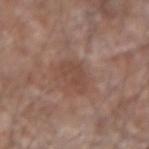Notes:
* notes: catalogued during a skin exam; not biopsied
* lighting: white-light illumination
* patient: male, about 60 years old
* anatomic site: the arm
* automated metrics: an average lesion color of about L≈46 a*≈19 b*≈26 (CIELAB) and a normalized lesion–skin contrast near 5.5; a within-lesion color-variation index near 1.5/10 and peripheral color asymmetry of about 0.5
* acquisition: 15 mm crop, total-body photography